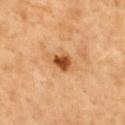Captured during whole-body skin photography for melanoma surveillance; the lesion was not biopsied. The total-body-photography lesion software estimated an automated nevus-likeness rating near 95 out of 100 and lesion-presence confidence of about 100/100. The subject is a male roughly 50 years of age. Imaged with cross-polarized lighting. The lesion is located on the mid back. A 15 mm crop from a total-body photograph taken for skin-cancer surveillance. Measured at roughly 2.5 mm in maximum diameter.